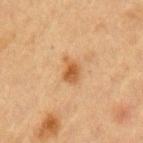Imaged during a routine full-body skin examination; the lesion was not biopsied and no histopathology is available. The lesion is located on the left upper arm. A region of skin cropped from a whole-body photographic capture, roughly 15 mm wide. A female patient aged 38 to 42.